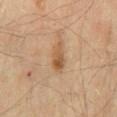The lesion was photographed on a routine skin check and not biopsied; there is no pathology result.
A male subject, in their mid-40s.
Cropped from a total-body skin-imaging series; the visible field is about 15 mm.
The lesion is on the back.